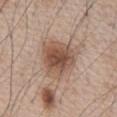Clinical impression:
The lesion was tiled from a total-body skin photograph and was not biopsied.
Clinical summary:
Located on the chest. Automated image analysis of the tile measured two-axis asymmetry of about 0.2. The analysis additionally found an average lesion color of about L≈51 a*≈18 b*≈27 (CIELAB) and about 12 CIELAB-L* units darker than the surrounding skin. Approximately 5 mm at its widest. A male subject approximately 65 years of age. A roughly 15 mm field-of-view crop from a total-body skin photograph.